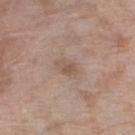Impression: Imaged during a routine full-body skin examination; the lesion was not biopsied and no histopathology is available. Acquisition and patient details: Cropped from a whole-body photographic skin survey; the tile spans about 15 mm. The patient is a female in their mid- to late 70s. Imaged with white-light lighting. The lesion is on the left lower leg.